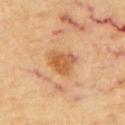acquisition = total-body-photography crop, ~15 mm field of view
subject = male, approximately 70 years of age
lesion size = ~4 mm (longest diameter)
site = the upper back
tile lighting = cross-polarized